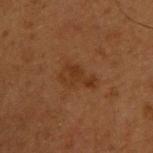Impression: Part of a total-body skin-imaging series; this lesion was reviewed on a skin check and was not flagged for biopsy. Image and clinical context: The recorded lesion diameter is about 4 mm. The tile uses cross-polarized illumination. A close-up tile cropped from a whole-body skin photograph, about 15 mm across. The subject is a male in their 50s. On the upper back.